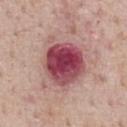Recorded during total-body skin imaging; not selected for excision or biopsy. The patient is a male approximately 75 years of age. A 15 mm close-up tile from a total-body photography series done for melanoma screening. From the chest. The lesion-visualizer software estimated an eccentricity of roughly 0.45 and a symmetry-axis asymmetry near 0.25. The tile uses white-light illumination. About 7 mm across.